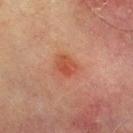Recorded during total-body skin imaging; not selected for excision or biopsy. Cropped from a whole-body photographic skin survey; the tile spans about 15 mm. The patient is a male aged 68–72. From the chest. Imaged with cross-polarized lighting.